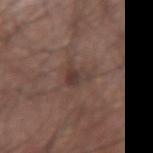Image and clinical context: The lesion's longest dimension is about 3.5 mm. A male subject, roughly 65 years of age. Automated tile analysis of the lesion measured a mean CIELAB color near L≈38 a*≈16 b*≈20, a lesion–skin lightness drop of about 7, and a lesion-to-skin contrast of about 6.5 (normalized; higher = more distinct). The analysis additionally found border irregularity of about 4.5 on a 0–10 scale and peripheral color asymmetry of about 1. This is a white-light tile. The lesion is on the arm. Cropped from a whole-body photographic skin survey; the tile spans about 15 mm.